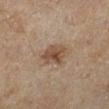This lesion was catalogued during total-body skin photography and was not selected for biopsy. On the left lower leg. A female subject, approximately 60 years of age. A 15 mm close-up tile from a total-body photography series done for melanoma screening. The lesion's longest dimension is about 3.5 mm. The lesion-visualizer software estimated a footprint of about 7 mm², an outline eccentricity of about 0.75 (0 = round, 1 = elongated), and two-axis asymmetry of about 0.3. It also reported a classifier nevus-likeness of about 60/100.